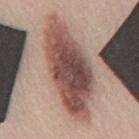Assessment:
This lesion was catalogued during total-body skin photography and was not selected for biopsy.
Image and clinical context:
A male patient, about 45 years old. On the mid back. This image is a 15 mm lesion crop taken from a total-body photograph.